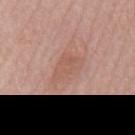notes: catalogued during a skin exam; not biopsied | tile lighting: white-light illumination | image: ~15 mm tile from a whole-body skin photo | patient: male, aged around 55 | lesion diameter: ≈5 mm | body site: the mid back.